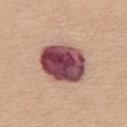follow-up = catalogued during a skin exam; not biopsied
site = the left thigh
image source = 15 mm crop, total-body photography
patient = female, aged approximately 60
diameter = ~5.5 mm (longest diameter)
illumination = white-light illumination